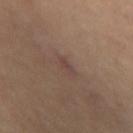No biopsy was performed on this lesion — it was imaged during a full skin examination and was not determined to be concerning. Imaged with cross-polarized lighting. The lesion's longest dimension is about 2.5 mm. The lesion is on the mid back. A close-up tile cropped from a whole-body skin photograph, about 15 mm across. Automated tile analysis of the lesion measured a mean CIELAB color near L≈43 a*≈17 b*≈21, a lesion–skin lightness drop of about 6, and a normalized border contrast of about 5.5. The analysis additionally found an automated nevus-likeness rating near 0 out of 100 and a detector confidence of about 80 out of 100 that the crop contains a lesion.